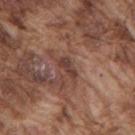Q: Was a biopsy performed?
A: imaged on a skin check; not biopsied
Q: Where on the body is the lesion?
A: the upper back
Q: How large is the lesion?
A: about 3 mm
Q: How was this image acquired?
A: total-body-photography crop, ~15 mm field of view
Q: What lighting was used for the tile?
A: white-light illumination
Q: Who is the patient?
A: male, aged approximately 75